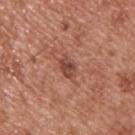Recorded during total-body skin imaging; not selected for excision or biopsy. Imaged with white-light lighting. A male subject aged around 55. From the back. Cropped from a whole-body photographic skin survey; the tile spans about 15 mm. Approximately 3 mm at its widest.